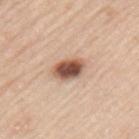illumination: white-light illumination | patient: male, roughly 45 years of age | diameter: about 4 mm | location: the upper back | imaging modality: 15 mm crop, total-body photography.